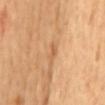Background:
A male patient approximately 65 years of age. The lesion is on the mid back. A region of skin cropped from a whole-body photographic capture, roughly 15 mm wide.